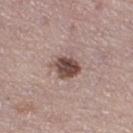Case summary:
– notes · imaged on a skin check; not biopsied
– anatomic site · the right thigh
– patient · female, approximately 50 years of age
– imaging modality · ~15 mm crop, total-body skin-cancer survey